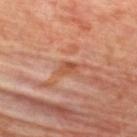This lesion was catalogued during total-body skin photography and was not selected for biopsy.
Approximately 2.5 mm at its widest.
Imaged with cross-polarized lighting.
Cropped from a whole-body photographic skin survey; the tile spans about 15 mm.
From the upper back.
The lesion-visualizer software estimated a footprint of about 3.5 mm² and an eccentricity of roughly 0.8. It also reported roughly 8 lightness units darker than nearby skin and a normalized lesion–skin contrast near 7. It also reported a nevus-likeness score of about 0/100 and a detector confidence of about 90 out of 100 that the crop contains a lesion.
A female subject aged around 45.